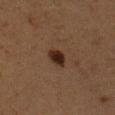Recorded during total-body skin imaging; not selected for excision or biopsy. A 15 mm crop from a total-body photograph taken for skin-cancer surveillance. Imaged with cross-polarized lighting. Measured at roughly 3 mm in maximum diameter. A female patient, approximately 50 years of age. On the left upper arm.